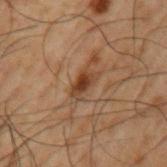Q: Was this lesion biopsied?
A: imaged on a skin check; not biopsied
Q: What kind of image is this?
A: ~15 mm crop, total-body skin-cancer survey
Q: Patient demographics?
A: male, aged 48–52
Q: Lesion location?
A: the left upper arm